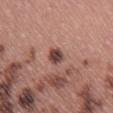Q: Was a biopsy performed?
A: catalogued during a skin exam; not biopsied
Q: Where on the body is the lesion?
A: the right thigh
Q: Illumination type?
A: white-light
Q: How was this image acquired?
A: total-body-photography crop, ~15 mm field of view
Q: Automated lesion metrics?
A: a mean CIELAB color near L≈44 a*≈21 b*≈22 and a lesion-to-skin contrast of about 11 (normalized; higher = more distinct); border irregularity of about 2 on a 0–10 scale, a color-variation rating of about 4.5/10, and a peripheral color-asymmetry measure near 1.5
Q: What is the lesion's diameter?
A: ≈2.5 mm
Q: Who is the patient?
A: female, aged 58 to 62